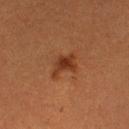notes=total-body-photography surveillance lesion; no biopsy
tile lighting=cross-polarized
subject=female, aged approximately 55
image source=15 mm crop, total-body photography
anatomic site=the left thigh
diameter=≈4 mm